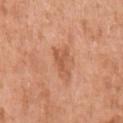Impression: The lesion was photographed on a routine skin check and not biopsied; there is no pathology result. Background: Automated tile analysis of the lesion measured a shape eccentricity near 0.8 and a symmetry-axis asymmetry near 0.4. And it measured a lesion color around L≈57 a*≈26 b*≈35 in CIELAB, roughly 9 lightness units darker than nearby skin, and a normalized lesion–skin contrast near 6. It also reported a border-irregularity index near 4.5/10, internal color variation of about 2 on a 0–10 scale, and radial color variation of about 0.5. It also reported a nevus-likeness score of about 0/100 and lesion-presence confidence of about 100/100. A lesion tile, about 15 mm wide, cut from a 3D total-body photograph. The tile uses white-light illumination. From the left upper arm. A female subject, aged approximately 65.